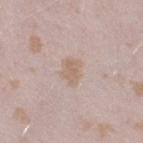| feature | finding |
|---|---|
| biopsy status | total-body-photography surveillance lesion; no biopsy |
| lesion diameter | ~3 mm (longest diameter) |
| image | ~15 mm crop, total-body skin-cancer survey |
| anatomic site | the right thigh |
| patient | female, in their mid-20s |